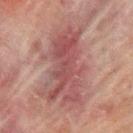{"biopsy_status": "not biopsied; imaged during a skin examination", "lighting": "cross-polarized", "automated_metrics": {"eccentricity": 0.85, "shape_asymmetry": 0.4, "cielab_L": 41, "cielab_a": 20, "cielab_b": 19, "vs_skin_darker_L": 8.0, "vs_skin_contrast_norm": 7.0, "nevus_likeness_0_100": 0, "lesion_detection_confidence_0_100": 95}, "image": {"source": "total-body photography crop", "field_of_view_mm": 15}, "lesion_size": {"long_diameter_mm_approx": 11.5}, "patient": {"sex": "male", "age_approx": 70}, "site": "back"}